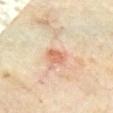workup = imaged on a skin check; not biopsied
image source = ~15 mm tile from a whole-body skin photo
size = ≈2.5 mm
location = the chest
subject = female, aged around 35